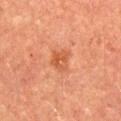The total-body-photography lesion software estimated a lesion area of about 5 mm², an eccentricity of roughly 0.45, and a symmetry-axis asymmetry near 0.3. The analysis additionally found a border-irregularity index near 3/10 and radial color variation of about 1.5. A male subject, aged 63 to 67. The recorded lesion diameter is about 2.5 mm. The lesion is on the abdomen. The tile uses cross-polarized illumination. A 15 mm close-up tile from a total-body photography series done for melanoma screening.